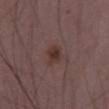The lesion was photographed on a routine skin check and not biopsied; there is no pathology result. Cropped from a whole-body photographic skin survey; the tile spans about 15 mm. Located on the left thigh. A male subject in their 50s.